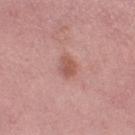Q: Was this lesion biopsied?
A: catalogued during a skin exam; not biopsied
Q: What are the patient's age and sex?
A: female, aged approximately 50
Q: What kind of image is this?
A: ~15 mm tile from a whole-body skin photo
Q: What is the lesion's diameter?
A: ~3 mm (longest diameter)
Q: Automated lesion metrics?
A: an area of roughly 4.5 mm², an outline eccentricity of about 0.7 (0 = round, 1 = elongated), and a shape-asymmetry score of about 0.2 (0 = symmetric); about 9 CIELAB-L* units darker than the surrounding skin and a lesion-to-skin contrast of about 6.5 (normalized; higher = more distinct)
Q: Illumination type?
A: white-light illumination
Q: What is the anatomic site?
A: the left thigh